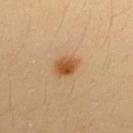Captured during whole-body skin photography for melanoma surveillance; the lesion was not biopsied. The tile uses cross-polarized illumination. Approximately 2.5 mm at its widest. A female subject aged 33–37. A lesion tile, about 15 mm wide, cut from a 3D total-body photograph. On the upper back.